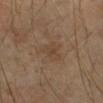Captured during whole-body skin photography for melanoma surveillance; the lesion was not biopsied. Cropped from a total-body skin-imaging series; the visible field is about 15 mm. Imaged with cross-polarized lighting. Located on the right forearm. Automated tile analysis of the lesion measured a mean CIELAB color near L≈36 a*≈13 b*≈25, roughly 5 lightness units darker than nearby skin, and a lesion-to-skin contrast of about 5 (normalized; higher = more distinct). A female subject, about 70 years old.